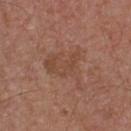Q: Is there a histopathology result?
A: total-body-photography surveillance lesion; no biopsy
Q: How large is the lesion?
A: about 5 mm
Q: Where on the body is the lesion?
A: the chest
Q: What did automated image analysis measure?
A: a lesion color around L≈46 a*≈21 b*≈28 in CIELAB, a lesion–skin lightness drop of about 6, and a normalized lesion–skin contrast near 5; a border-irregularity index near 7/10, a within-lesion color-variation index near 2.5/10, and radial color variation of about 0.5; a nevus-likeness score of about 0/100
Q: Illumination type?
A: white-light
Q: What kind of image is this?
A: 15 mm crop, total-body photography
Q: Patient demographics?
A: male, aged approximately 55